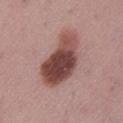| field | value |
|---|---|
| biopsy status | catalogued during a skin exam; not biopsied |
| image | ~15 mm crop, total-body skin-cancer survey |
| patient | female, in their mid- to late 50s |
| automated metrics | two-axis asymmetry of about 0.3; a mean CIELAB color near L≈46 a*≈23 b*≈22, roughly 17 lightness units darker than nearby skin, and a normalized lesion–skin contrast near 12; border irregularity of about 3 on a 0–10 scale and a peripheral color-asymmetry measure near 2.5 |
| size | ~7.5 mm (longest diameter) |
| tile lighting | white-light |
| body site | the left lower leg |